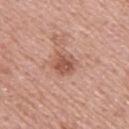Imaged during a routine full-body skin examination; the lesion was not biopsied and no histopathology is available.
The patient is a male aged 53–57.
A 15 mm close-up tile from a total-body photography series done for melanoma screening.
On the upper back.